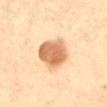biopsy status — no biopsy performed (imaged during a skin exam) | location — the abdomen | patient — female, about 65 years old | illumination — cross-polarized illumination | diameter — about 4.5 mm | image — ~15 mm tile from a whole-body skin photo | TBP lesion metrics — a footprint of about 15 mm², an outline eccentricity of about 0.4 (0 = round, 1 = elongated), and two-axis asymmetry of about 0.2; a mean CIELAB color near L≈67 a*≈22 b*≈38, about 16 CIELAB-L* units darker than the surrounding skin, and a lesion-to-skin contrast of about 9 (normalized; higher = more distinct); a detector confidence of about 100 out of 100 that the crop contains a lesion.